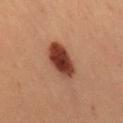Q: Was this lesion biopsied?
A: total-body-photography surveillance lesion; no biopsy
Q: Illumination type?
A: cross-polarized
Q: What is the anatomic site?
A: the right thigh
Q: How was this image acquired?
A: 15 mm crop, total-body photography
Q: What did automated image analysis measure?
A: a lesion area of about 12 mm², a shape eccentricity near 0.85, and two-axis asymmetry of about 0.1; a lesion color around L≈41 a*≈26 b*≈30 in CIELAB, roughly 17 lightness units darker than nearby skin, and a lesion-to-skin contrast of about 13 (normalized; higher = more distinct); border irregularity of about 1.5 on a 0–10 scale, a color-variation rating of about 6/10, and peripheral color asymmetry of about 2
Q: Patient demographics?
A: male, aged 48–52